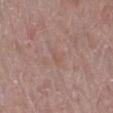{"biopsy_status": "not biopsied; imaged during a skin examination", "image": {"source": "total-body photography crop", "field_of_view_mm": 15}, "patient": {"sex": "female", "age_approx": 60}, "site": "leg"}